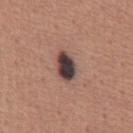workup: total-body-photography surveillance lesion; no biopsy | illumination: white-light illumination | subject: male, about 45 years old | size: ≈4 mm | automated lesion analysis: a lesion area of about 7 mm², a shape eccentricity near 0.8, and a shape-asymmetry score of about 0.15 (0 = symmetric); an average lesion color of about L≈40 a*≈15 b*≈18 (CIELAB), roughly 19 lightness units darker than nearby skin, and a normalized border contrast of about 15.5; border irregularity of about 1.5 on a 0–10 scale, internal color variation of about 7.5 on a 0–10 scale, and a peripheral color-asymmetry measure near 2.5 | anatomic site: the abdomen | image source: total-body-photography crop, ~15 mm field of view.